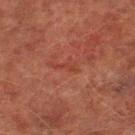Clinical impression: The lesion was tiled from a total-body skin photograph and was not biopsied. Context: Captured under cross-polarized illumination. A close-up tile cropped from a whole-body skin photograph, about 15 mm across. The lesion is located on the leg. A male subject, about 75 years old. Measured at roughly 3 mm in maximum diameter.